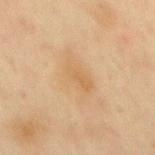notes: total-body-photography surveillance lesion; no biopsy | image-analysis metrics: an area of roughly 11 mm² and an eccentricity of roughly 0.9; about 5 CIELAB-L* units darker than the surrounding skin and a lesion-to-skin contrast of about 4.5 (normalized; higher = more distinct); a border-irregularity rating of about 4/10, internal color variation of about 2.5 on a 0–10 scale, and peripheral color asymmetry of about 0.5; a nevus-likeness score of about 0/100 and lesion-presence confidence of about 100/100 | tile lighting: cross-polarized illumination | lesion size: ≈5.5 mm | patient: male, in their mid-40s | site: the mid back | image: 15 mm crop, total-body photography.